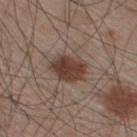biopsy status: imaged on a skin check; not biopsied
subject: male, approximately 60 years of age
imaging modality: total-body-photography crop, ~15 mm field of view
site: the left lower leg